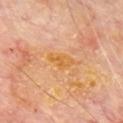Recorded during total-body skin imaging; not selected for excision or biopsy.
Imaged with cross-polarized lighting.
The total-body-photography lesion software estimated a lesion area of about 2.5 mm², a shape eccentricity near 0.85, and a symmetry-axis asymmetry near 0.6. The software also gave an average lesion color of about L≈63 a*≈24 b*≈49 (CIELAB), roughly 7 lightness units darker than nearby skin, and a normalized border contrast of about 8. And it measured an automated nevus-likeness rating near 0 out of 100 and a detector confidence of about 100 out of 100 that the crop contains a lesion.
The lesion is on the chest.
Approximately 3 mm at its widest.
A 15 mm crop from a total-body photograph taken for skin-cancer surveillance.
A male patient, roughly 65 years of age.